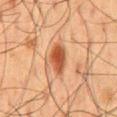Q: What are the patient's age and sex?
A: male, roughly 60 years of age
Q: What is the imaging modality?
A: ~15 mm tile from a whole-body skin photo
Q: Lesion location?
A: the mid back